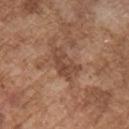lighting = white-light
imaging modality = 15 mm crop, total-body photography
subject = male, aged 73–77
location = the chest
automated metrics = an area of roughly 7.5 mm² and a symmetry-axis asymmetry near 0.35; about 9 CIELAB-L* units darker than the surrounding skin and a normalized border contrast of about 7; a border-irregularity index near 4/10 and internal color variation of about 3.5 on a 0–10 scale; a lesion-detection confidence of about 95/100
diameter = ≈4 mm